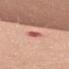  biopsy_status: not biopsied; imaged during a skin examination